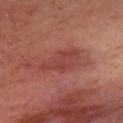notes: no biopsy performed (imaged during a skin exam); image source: ~15 mm tile from a whole-body skin photo; illumination: cross-polarized illumination; patient: female, aged around 40; location: the left upper arm.